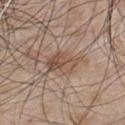Assessment: Part of a total-body skin-imaging series; this lesion was reviewed on a skin check and was not flagged for biopsy. Context: The subject is a male aged around 50. On the front of the torso. A 15 mm close-up extracted from a 3D total-body photography capture. Approximately 4.5 mm at its widest. An algorithmic analysis of the crop reported a lesion color around L≈51 a*≈16 b*≈28 in CIELAB, a lesion–skin lightness drop of about 9, and a normalized border contrast of about 7. And it measured border irregularity of about 4 on a 0–10 scale and peripheral color asymmetry of about 2.5. And it measured a nevus-likeness score of about 30/100 and lesion-presence confidence of about 100/100.